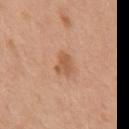Clinical impression: The lesion was photographed on a routine skin check and not biopsied; there is no pathology result. Acquisition and patient details: A close-up tile cropped from a whole-body skin photograph, about 15 mm across. From the upper back. Captured under white-light illumination. About 3 mm across. Automated image analysis of the tile measured a lesion-detection confidence of about 100/100. The patient is a male in their mid-50s.